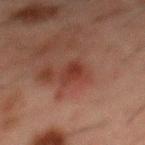Notes:
- workup: total-body-photography surveillance lesion; no biopsy
- acquisition: ~15 mm crop, total-body skin-cancer survey
- location: the mid back
- automated lesion analysis: a lesion–skin lightness drop of about 7 and a normalized lesion–skin contrast near 7.5; border irregularity of about 3 on a 0–10 scale and radial color variation of about 0.5; a classifier nevus-likeness of about 0/100 and a detector confidence of about 100 out of 100 that the crop contains a lesion
- illumination: cross-polarized illumination
- diameter: about 3 mm
- subject: male, in their 50s